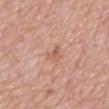Impression:
This lesion was catalogued during total-body skin photography and was not selected for biopsy.
Context:
A region of skin cropped from a whole-body photographic capture, roughly 15 mm wide. The total-body-photography lesion software estimated an automated nevus-likeness rating near 0 out of 100 and a detector confidence of about 100 out of 100 that the crop contains a lesion. The lesion is on the mid back. A male patient, in their mid- to late 70s. The recorded lesion diameter is about 2.5 mm.